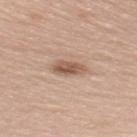| feature | finding |
|---|---|
| workup | imaged on a skin check; not biopsied |
| diameter | about 3.5 mm |
| image | ~15 mm tile from a whole-body skin photo |
| body site | the upper back |
| lighting | white-light illumination |
| subject | female, about 60 years old |